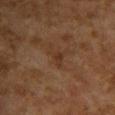Clinical impression:
This lesion was catalogued during total-body skin photography and was not selected for biopsy.
Clinical summary:
Automated tile analysis of the lesion measured a shape eccentricity near 0.85 and two-axis asymmetry of about 0.45. The software also gave a mean CIELAB color near L≈29 a*≈16 b*≈26, roughly 5 lightness units darker than nearby skin, and a normalized lesion–skin contrast near 6. And it measured a border-irregularity rating of about 5/10. The patient is a female aged approximately 60. This is a cross-polarized tile. The lesion's longest dimension is about 3 mm. A lesion tile, about 15 mm wide, cut from a 3D total-body photograph. The lesion is located on the back.